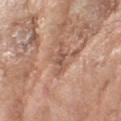The lesion was photographed on a routine skin check and not biopsied; there is no pathology result. The recorded lesion diameter is about 3 mm. A roughly 15 mm field-of-view crop from a total-body skin photograph. Located on the left forearm. The subject is a female aged around 75. The lesion-visualizer software estimated a footprint of about 3 mm², an outline eccentricity of about 0.9 (0 = round, 1 = elongated), and a symmetry-axis asymmetry near 0.35. It also reported a border-irregularity rating of about 4/10, internal color variation of about 1.5 on a 0–10 scale, and peripheral color asymmetry of about 0.5. The software also gave a nevus-likeness score of about 0/100 and lesion-presence confidence of about 85/100.